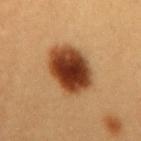| feature | finding |
|---|---|
| biopsy status | imaged on a skin check; not biopsied |
| acquisition | ~15 mm crop, total-body skin-cancer survey |
| subject | female, aged 28–32 |
| body site | the mid back |